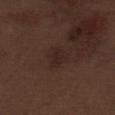biopsy status: total-body-photography surveillance lesion; no biopsy | subject: male, approximately 70 years of age | acquisition: 15 mm crop, total-body photography | body site: the abdomen | size: about 3 mm.